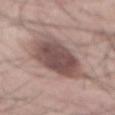biopsy status = catalogued during a skin exam; not biopsied | site = the mid back | imaging modality = total-body-photography crop, ~15 mm field of view | lighting = white-light illumination | subject = male, about 55 years old | diameter = ~9 mm (longest diameter).